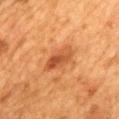| field | value |
|---|---|
| biopsy status | total-body-photography surveillance lesion; no biopsy |
| location | the mid back |
| image source | 15 mm crop, total-body photography |
| automated lesion analysis | a shape eccentricity near 0.9 and a symmetry-axis asymmetry near 0.25; a lesion color around L≈44 a*≈25 b*≈37 in CIELAB, a lesion–skin lightness drop of about 10, and a normalized lesion–skin contrast near 7.5; a nevus-likeness score of about 55/100 |
| patient | female, about 55 years old |
| illumination | cross-polarized |
| lesion diameter | ≈4.5 mm |